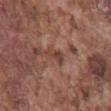Assessment:
No biopsy was performed on this lesion — it was imaged during a full skin examination and was not determined to be concerning.
Image and clinical context:
A lesion tile, about 15 mm wide, cut from a 3D total-body photograph. Located on the mid back. A male subject, roughly 75 years of age.